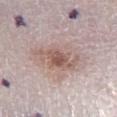* tile lighting · white-light
* site · the right lower leg
* size · ≈7 mm
* patient · male, approximately 50 years of age
* acquisition · ~15 mm crop, total-body skin-cancer survey
* automated lesion analysis · a lesion color around L≈60 a*≈16 b*≈22 in CIELAB and a normalized border contrast of about 6.5; an automated nevus-likeness rating near 65 out of 100 and lesion-presence confidence of about 100/100